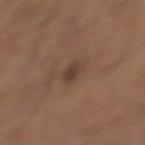notes = catalogued during a skin exam; not biopsied
body site = the left lower leg
imaging modality = 15 mm crop, total-body photography
patient = male, roughly 30 years of age
lesion size = ≈3 mm